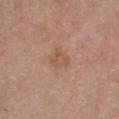biopsy status: catalogued during a skin exam; not biopsied
subject: female, aged 48 to 52
site: the chest
automated lesion analysis: border irregularity of about 2.5 on a 0–10 scale, internal color variation of about 2 on a 0–10 scale, and radial color variation of about 0.5; a nevus-likeness score of about 0/100 and a lesion-detection confidence of about 100/100
illumination: white-light
image source: ~15 mm crop, total-body skin-cancer survey
size: ~3 mm (longest diameter)